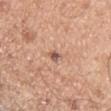{"biopsy_status": "not biopsied; imaged during a skin examination", "image": {"source": "total-body photography crop", "field_of_view_mm": 15}, "patient": {"sex": "male", "age_approx": 55}, "site": "left upper arm"}